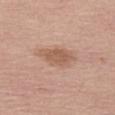Assessment: Part of a total-body skin-imaging series; this lesion was reviewed on a skin check and was not flagged for biopsy.